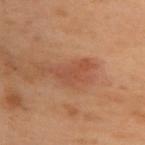Q: Was a biopsy performed?
A: total-body-photography surveillance lesion; no biopsy
Q: What is the imaging modality?
A: total-body-photography crop, ~15 mm field of view
Q: Lesion size?
A: ~4.5 mm (longest diameter)
Q: Lesion location?
A: the back
Q: Automated lesion metrics?
A: an area of roughly 6.5 mm², an eccentricity of roughly 0.9, and a shape-asymmetry score of about 0.6 (0 = symmetric); an average lesion color of about L≈41 a*≈21 b*≈28 (CIELAB), about 7 CIELAB-L* units darker than the surrounding skin, and a lesion-to-skin contrast of about 6 (normalized; higher = more distinct); lesion-presence confidence of about 100/100
Q: How was the tile lit?
A: cross-polarized
Q: What are the patient's age and sex?
A: female, aged around 55